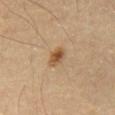Recorded during total-body skin imaging; not selected for excision or biopsy. A close-up tile cropped from a whole-body skin photograph, about 15 mm across. Automated tile analysis of the lesion measured a symmetry-axis asymmetry near 0.25. The analysis additionally found border irregularity of about 2 on a 0–10 scale, a within-lesion color-variation index near 4.5/10, and a peripheral color-asymmetry measure near 1.5. The lesion is on the chest. The subject is a male aged around 55. Imaged with cross-polarized lighting. The lesion's longest dimension is about 2.5 mm.